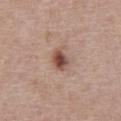This lesion was catalogued during total-body skin photography and was not selected for biopsy.
A male patient, aged 58–62.
A 15 mm close-up extracted from a 3D total-body photography capture.
This is a white-light tile.
Longest diameter approximately 3 mm.
The lesion-visualizer software estimated a border-irregularity index near 2/10, a color-variation rating of about 6.5/10, and a peripheral color-asymmetry measure near 2. The software also gave a nevus-likeness score of about 95/100 and lesion-presence confidence of about 100/100.
The lesion is on the chest.